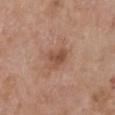image-analysis metrics: roughly 9 lightness units darker than nearby skin; border irregularity of about 3 on a 0–10 scale, internal color variation of about 2.5 on a 0–10 scale, and peripheral color asymmetry of about 1; a classifier nevus-likeness of about 10/100 | location: the chest | lighting: white-light | image source: 15 mm crop, total-body photography | patient: female, in their mid-60s | diameter: ~3 mm (longest diameter).